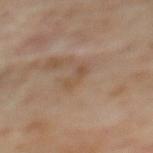The lesion is located on the upper back. The tile uses cross-polarized illumination. Cropped from a whole-body photographic skin survey; the tile spans about 15 mm. A female patient, in their 60s. Approximately 3 mm at its widest. Automated image analysis of the tile measured a shape eccentricity near 0.9 and a symmetry-axis asymmetry near 0.4. The software also gave an average lesion color of about L≈50 a*≈16 b*≈29 (CIELAB) and a lesion-to-skin contrast of about 5 (normalized; higher = more distinct). The analysis additionally found radial color variation of about 0. It also reported a classifier nevus-likeness of about 0/100 and a detector confidence of about 100 out of 100 that the crop contains a lesion.